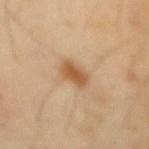Captured during whole-body skin photography for melanoma surveillance; the lesion was not biopsied.
The lesion is on the mid back.
Captured under cross-polarized illumination.
Measured at roughly 3 mm in maximum diameter.
The subject is a male in their 40s.
Cropped from a whole-body photographic skin survey; the tile spans about 15 mm.